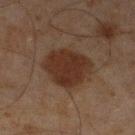  biopsy_status: not biopsied; imaged during a skin examination
  lighting: cross-polarized
  patient:
    sex: male
    age_approx: 45
  image:
    source: total-body photography crop
    field_of_view_mm: 15
  site: left lower leg
  automated_metrics:
    area_mm2_approx: 20.0
    eccentricity: 0.45
    shape_asymmetry: 0.15
    nevus_likeness_0_100: 65
    lesion_detection_confidence_0_100: 100